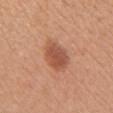follow-up: catalogued during a skin exam; not biopsied
imaging modality: total-body-photography crop, ~15 mm field of view
subject: female, roughly 45 years of age
anatomic site: the chest
illumination: white-light illumination
TBP lesion metrics: a border-irregularity rating of about 2/10, internal color variation of about 4 on a 0–10 scale, and radial color variation of about 1.5; a lesion-detection confidence of about 100/100
lesion diameter: ~4 mm (longest diameter)